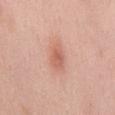The lesion was tiled from a total-body skin photograph and was not biopsied.
From the mid back.
The subject is a female roughly 50 years of age.
This image is a 15 mm lesion crop taken from a total-body photograph.